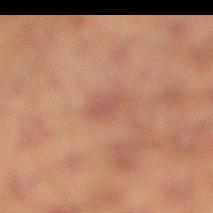Impression:
The lesion was photographed on a routine skin check and not biopsied; there is no pathology result.
Image and clinical context:
A male patient, aged 43 to 47. Cropped from a total-body skin-imaging series; the visible field is about 15 mm. The lesion's longest dimension is about 3 mm. Located on the right lower leg. Imaged with cross-polarized lighting.